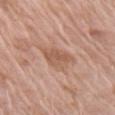biopsy status = no biopsy performed (imaged during a skin exam).